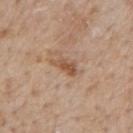Captured during whole-body skin photography for melanoma surveillance; the lesion was not biopsied. A 15 mm crop from a total-body photograph taken for skin-cancer surveillance. The lesion is on the mid back. The patient is a male in their mid-60s. Captured under white-light illumination.